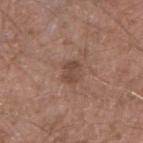| field | value |
|---|---|
| follow-up | total-body-photography surveillance lesion; no biopsy |
| lesion size | ~2.5 mm (longest diameter) |
| image source | 15 mm crop, total-body photography |
| patient | male, in their mid-50s |
| automated metrics | a lesion-detection confidence of about 100/100 |
| site | the arm |
| illumination | white-light |